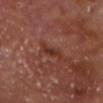workup: no biopsy performed (imaged during a skin exam) | anatomic site: the head or neck | illumination: cross-polarized | patient: male, in their mid-60s | image: total-body-photography crop, ~15 mm field of view | TBP lesion metrics: an area of roughly 3 mm² and a symmetry-axis asymmetry near 0.4; a border-irregularity rating of about 4/10, a within-lesion color-variation index near 0.5/10, and peripheral color asymmetry of about 0.5.